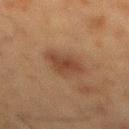This lesion was catalogued during total-body skin photography and was not selected for biopsy. On the mid back. A male patient, about 60 years old. Cropped from a whole-body photographic skin survey; the tile spans about 15 mm. Imaged with cross-polarized lighting. About 4 mm across. The lesion-visualizer software estimated a lesion area of about 9 mm², a shape eccentricity near 0.65, and two-axis asymmetry of about 0.3. The software also gave a border-irregularity rating of about 3/10, internal color variation of about 2.5 on a 0–10 scale, and radial color variation of about 1. It also reported an automated nevus-likeness rating near 55 out of 100 and lesion-presence confidence of about 100/100.